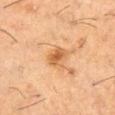<lesion>
  <biopsy_status>not biopsied; imaged during a skin examination</biopsy_status>
  <lighting>cross-polarized</lighting>
  <site>leg</site>
  <patient>
    <sex>male</sex>
    <age_approx>60</age_approx>
  </patient>
  <image>
    <source>total-body photography crop</source>
    <field_of_view_mm>15</field_of_view_mm>
  </image>
  <lesion_size>
    <long_diameter_mm_approx>3.0</long_diameter_mm_approx>
  </lesion_size>
  <automated_metrics>
    <cielab_L>59</cielab_L>
    <cielab_a>23</cielab_a>
    <cielab_b>41</cielab_b>
    <vs_skin_darker_L>10.0</vs_skin_darker_L>
    <vs_skin_contrast_norm>7.0</vs_skin_contrast_norm>
    <color_variation_0_10>5.5</color_variation_0_10>
    <peripheral_color_asymmetry>1.5</peripheral_color_asymmetry>
    <lesion_detection_confidence_0_100>100</lesion_detection_confidence_0_100>
  </automated_metrics>
</lesion>